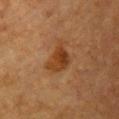biopsy_status: not biopsied; imaged during a skin examination
lesion_size:
  long_diameter_mm_approx: 3.5
lighting: cross-polarized
image:
  source: total-body photography crop
  field_of_view_mm: 15
patient:
  sex: female
  age_approx: 55
site: chest